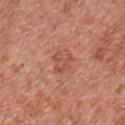notes — no biopsy performed (imaged during a skin exam) | automated lesion analysis — an area of roughly 4.5 mm², a shape eccentricity near 0.2, and a shape-asymmetry score of about 0.5 (0 = symmetric) | patient — male, roughly 70 years of age | image — 15 mm crop, total-body photography | body site — the chest.